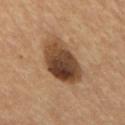workup — no biopsy performed (imaged during a skin exam)
lesion size — about 6 mm
body site — the back
lighting — cross-polarized illumination
image source — total-body-photography crop, ~15 mm field of view
automated lesion analysis — a mean CIELAB color near L≈40 a*≈17 b*≈29, a lesion–skin lightness drop of about 16, and a lesion-to-skin contrast of about 12.5 (normalized; higher = more distinct); a classifier nevus-likeness of about 60/100 and lesion-presence confidence of about 100/100
patient — male, in their mid- to late 50s